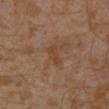Captured during whole-body skin photography for melanoma surveillance; the lesion was not biopsied.
Cropped from a whole-body photographic skin survey; the tile spans about 15 mm.
A male subject aged approximately 30.
The tile uses cross-polarized illumination.
On the left lower leg.